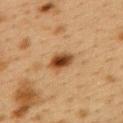Recorded during total-body skin imaging; not selected for excision or biopsy.
Captured under cross-polarized illumination.
A female patient aged around 40.
A 15 mm close-up extracted from a 3D total-body photography capture.
Measured at roughly 3.5 mm in maximum diameter.
On the upper back.